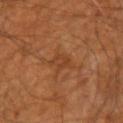workup = no biopsy performed (imaged during a skin exam) | illumination = cross-polarized | subject = male, aged 58–62 | lesion diameter = ≈3 mm | imaging modality = ~15 mm crop, total-body skin-cancer survey | site = the arm | image-analysis metrics = an eccentricity of roughly 0.85; a lesion color around L≈40 a*≈23 b*≈35 in CIELAB and about 6 CIELAB-L* units darker than the surrounding skin; a within-lesion color-variation index near 3/10 and radial color variation of about 1; an automated nevus-likeness rating near 0 out of 100.